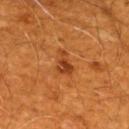A male patient, aged around 60.
A region of skin cropped from a whole-body photographic capture, roughly 15 mm wide.
The lesion is on the mid back.
The recorded lesion diameter is about 3 mm.